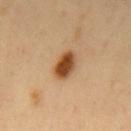Imaged during a routine full-body skin examination; the lesion was not biopsied and no histopathology is available. A male subject, aged 48 to 52. The lesion is on the mid back. A 15 mm close-up extracted from a 3D total-body photography capture.